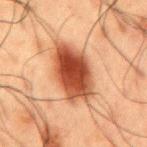biopsy status — total-body-photography surveillance lesion; no biopsy
subject — male, aged approximately 60
anatomic site — the mid back
image source — ~15 mm tile from a whole-body skin photo